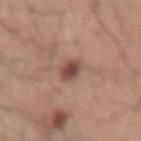subject = male, aged approximately 60; site = the right forearm; image source = 15 mm crop, total-body photography; size = about 2.5 mm; tile lighting = white-light.